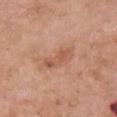Q: Is there a histopathology result?
A: total-body-photography surveillance lesion; no biopsy
Q: How was the tile lit?
A: white-light illumination
Q: How was this image acquired?
A: total-body-photography crop, ~15 mm field of view
Q: How large is the lesion?
A: ≈4.5 mm
Q: What is the anatomic site?
A: the chest
Q: Who is the patient?
A: female, in their 60s